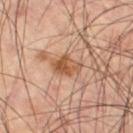Q: Was this lesion biopsied?
A: no biopsy performed (imaged during a skin exam)
Q: What are the patient's age and sex?
A: male, roughly 60 years of age
Q: What is the imaging modality?
A: 15 mm crop, total-body photography
Q: How was the tile lit?
A: cross-polarized illumination
Q: Where on the body is the lesion?
A: the right thigh